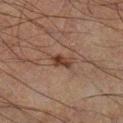notes: imaged on a skin check; not biopsied
automated lesion analysis: an area of roughly 3 mm², a shape eccentricity near 0.85, and two-axis asymmetry of about 0.35; a mean CIELAB color near L≈29 a*≈16 b*≈22 and a lesion–skin lightness drop of about 9; border irregularity of about 3 on a 0–10 scale, a color-variation rating of about 1.5/10, and radial color variation of about 0.5; an automated nevus-likeness rating near 90 out of 100 and a lesion-detection confidence of about 100/100
body site: the right lower leg
image source: total-body-photography crop, ~15 mm field of view
patient: male, aged approximately 50